A roughly 15 mm field-of-view crop from a total-body skin photograph. The lesion is located on the head or neck. A male subject, aged 78 to 82 — 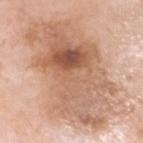Conclusion: Histopathological examination showed a skin cancer: melanoma in situ, lentigo maligna type.